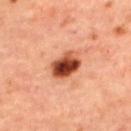Case summary:
• follow-up · catalogued during a skin exam; not biopsied
• image · total-body-photography crop, ~15 mm field of view
• illumination · cross-polarized illumination
• site · the back
• subject · female, in their mid- to late 40s
• lesion size · ~4 mm (longest diameter)